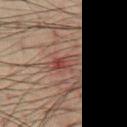| field | value |
|---|---|
| biopsy status | total-body-photography surveillance lesion; no biopsy |
| body site | the chest |
| diameter | ~3.5 mm (longest diameter) |
| tile lighting | cross-polarized |
| acquisition | total-body-photography crop, ~15 mm field of view |
| subject | male, aged approximately 60 |